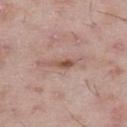Case summary:
* image source — ~15 mm crop, total-body skin-cancer survey
* automated metrics — an eccentricity of roughly 0.95 and a symmetry-axis asymmetry near 0.35; roughly 11 lightness units darker than nearby skin and a normalized border contrast of about 8; a within-lesion color-variation index near 0/10 and peripheral color asymmetry of about 0
* site — the right thigh
* size — ~3 mm (longest diameter)
* subject — male, in their 50s